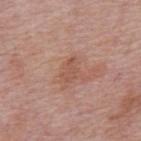Impression: Imaged during a routine full-body skin examination; the lesion was not biopsied and no histopathology is available. Clinical summary: This image is a 15 mm lesion crop taken from a total-body photograph. A male patient, in their mid-70s. The lesion-visualizer software estimated an area of roughly 3.5 mm². It also reported a classifier nevus-likeness of about 0/100. Measured at roughly 3 mm in maximum diameter. This is a white-light tile. Located on the mid back.